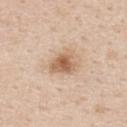Recorded during total-body skin imaging; not selected for excision or biopsy. About 3.5 mm across. The subject is a female aged 43 to 47. An algorithmic analysis of the crop reported an automated nevus-likeness rating near 85 out of 100. On the upper back. Cropped from a total-body skin-imaging series; the visible field is about 15 mm.